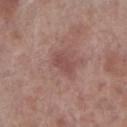| key | value |
|---|---|
| notes | no biopsy performed (imaged during a skin exam) |
| acquisition | total-body-photography crop, ~15 mm field of view |
| subject | male, approximately 70 years of age |
| location | the right lower leg |
| automated metrics | a footprint of about 4 mm², an outline eccentricity of about 0.85 (0 = round, 1 = elongated), and two-axis asymmetry of about 0.25; an average lesion color of about L≈48 a*≈22 b*≈22 (CIELAB), about 7 CIELAB-L* units darker than the surrounding skin, and a lesion-to-skin contrast of about 5.5 (normalized; higher = more distinct); a classifier nevus-likeness of about 0/100 and lesion-presence confidence of about 100/100 |
| illumination | white-light |